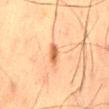{
  "biopsy_status": "not biopsied; imaged during a skin examination",
  "patient": {
    "sex": "male",
    "age_approx": 60
  },
  "site": "mid back",
  "image": {
    "source": "total-body photography crop",
    "field_of_view_mm": 15
  }
}